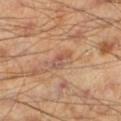Findings:
– imaging modality — total-body-photography crop, ~15 mm field of view
– subject — male, in their mid-40s
– anatomic site — the left lower leg
– illumination — cross-polarized illumination